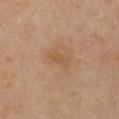Imaged during a routine full-body skin examination; the lesion was not biopsied and no histopathology is available. A female subject, aged 38 to 42. Cropped from a whole-body photographic skin survey; the tile spans about 15 mm. The lesion is on the chest.